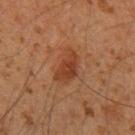Recorded during total-body skin imaging; not selected for excision or biopsy.